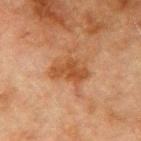An algorithmic analysis of the crop reported border irregularity of about 3 on a 0–10 scale, internal color variation of about 3.5 on a 0–10 scale, and radial color variation of about 1. The analysis additionally found a classifier nevus-likeness of about 20/100 and a detector confidence of about 100 out of 100 that the crop contains a lesion.
A male patient roughly 75 years of age.
A lesion tile, about 15 mm wide, cut from a 3D total-body photograph.
On the right upper arm.
Imaged with cross-polarized lighting.